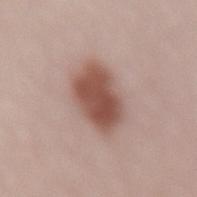Impression: Part of a total-body skin-imaging series; this lesion was reviewed on a skin check and was not flagged for biopsy. Background: An algorithmic analysis of the crop reported a footprint of about 16 mm², an outline eccentricity of about 0.8 (0 = round, 1 = elongated), and a shape-asymmetry score of about 0.15 (0 = symmetric). It also reported a lesion-to-skin contrast of about 10 (normalized; higher = more distinct). It also reported a nevus-likeness score of about 100/100. A 15 mm close-up tile from a total-body photography series done for melanoma screening. Measured at roughly 5.5 mm in maximum diameter. Captured under white-light illumination. A female subject aged around 40. The lesion is on the lower back.